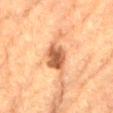A region of skin cropped from a whole-body photographic capture, roughly 15 mm wide. A male patient roughly 85 years of age or older. The lesion is on the lower back. Automated image analysis of the tile measured an average lesion color of about L≈52 a*≈24 b*≈35 (CIELAB) and roughly 16 lightness units darker than nearby skin. It also reported a border-irregularity index near 2.5/10, a color-variation rating of about 5/10, and peripheral color asymmetry of about 1.5. And it measured a lesion-detection confidence of about 100/100.